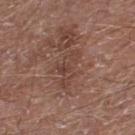  biopsy_status: not biopsied; imaged during a skin examination
  site: leg
  lighting: white-light
  patient:
    sex: male
    age_approx: 70
  image:
    source: total-body photography crop
    field_of_view_mm: 15